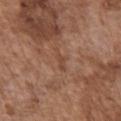Clinical impression: The lesion was photographed on a routine skin check and not biopsied; there is no pathology result. Acquisition and patient details: A male patient, about 75 years old. About 3.5 mm across. From the front of the torso. A 15 mm close-up extracted from a 3D total-body photography capture.